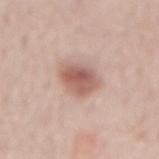| feature | finding |
|---|---|
| biopsy status | imaged on a skin check; not biopsied |